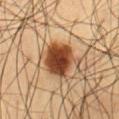The lesion was photographed on a routine skin check and not biopsied; there is no pathology result.
Imaged with cross-polarized lighting.
A close-up tile cropped from a whole-body skin photograph, about 15 mm across.
The subject is a male approximately 60 years of age.
Automated tile analysis of the lesion measured an area of roughly 14 mm² and two-axis asymmetry of about 0.2. It also reported a border-irregularity index near 2.5/10 and a peripheral color-asymmetry measure near 1.5. And it measured lesion-presence confidence of about 100/100.
Located on the abdomen.